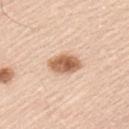{"biopsy_status": "not biopsied; imaged during a skin examination", "automated_metrics": {"cielab_L": 63, "cielab_a": 20, "cielab_b": 33, "vs_skin_darker_L": 16.0, "vs_skin_contrast_norm": 9.5}, "lesion_size": {"long_diameter_mm_approx": 4.0}, "site": "left upper arm", "image": {"source": "total-body photography crop", "field_of_view_mm": 15}, "lighting": "white-light", "patient": {"sex": "male", "age_approx": 45}}